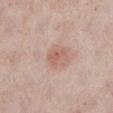Q: Is there a histopathology result?
A: no biopsy performed (imaged during a skin exam)
Q: Illumination type?
A: white-light
Q: Patient demographics?
A: female, aged 58–62
Q: What kind of image is this?
A: ~15 mm tile from a whole-body skin photo
Q: Lesion location?
A: the left lower leg